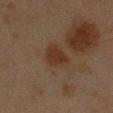Part of a total-body skin-imaging series; this lesion was reviewed on a skin check and was not flagged for biopsy.
A close-up tile cropped from a whole-body skin photograph, about 15 mm across.
Approximately 3.5 mm at its widest.
From the left forearm.
Imaged with cross-polarized lighting.
The patient is a male aged 43–47.
The lesion-visualizer software estimated a lesion–skin lightness drop of about 6. The analysis additionally found a border-irregularity rating of about 2.5/10, a within-lesion color-variation index near 2/10, and peripheral color asymmetry of about 1.